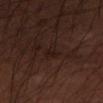Impression:
Part of a total-body skin-imaging series; this lesion was reviewed on a skin check and was not flagged for biopsy.
Clinical summary:
A region of skin cropped from a whole-body photographic capture, roughly 15 mm wide. A male subject aged around 50. Approximately 3 mm at its widest. Captured under cross-polarized illumination. The lesion is located on the arm.